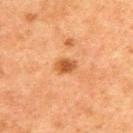follow-up = total-body-photography surveillance lesion; no biopsy
acquisition = ~15 mm tile from a whole-body skin photo
anatomic site = the back
patient = male, approximately 50 years of age